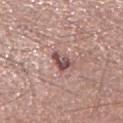biopsy status: total-body-photography surveillance lesion; no biopsy
image source: ~15 mm tile from a whole-body skin photo
illumination: white-light
size: ~3 mm (longest diameter)
subject: male, aged 53 to 57
location: the right lower leg
automated metrics: a footprint of about 4.5 mm², an outline eccentricity of about 0.75 (0 = round, 1 = elongated), and two-axis asymmetry of about 0.3; a border-irregularity index near 3/10 and a color-variation rating of about 5/10; a classifier nevus-likeness of about 0/100 and lesion-presence confidence of about 100/100